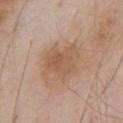| key | value |
|---|---|
| notes | imaged on a skin check; not biopsied |
| subject | male, roughly 55 years of age |
| tile lighting | white-light illumination |
| lesion diameter | ~4 mm (longest diameter) |
| image | 15 mm crop, total-body photography |
| anatomic site | the chest |